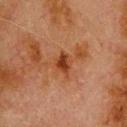Case summary:
* notes — no biopsy performed (imaged during a skin exam)
* illumination — cross-polarized
* patient — male, aged 78 to 82
* body site — the back
* image — total-body-photography crop, ~15 mm field of view